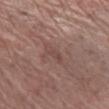Assessment:
This lesion was catalogued during total-body skin photography and was not selected for biopsy.
Clinical summary:
A male subject in their 70s. Imaged with white-light lighting. Approximately 3.5 mm at its widest. Located on the right lower leg. A 15 mm close-up extracted from a 3D total-body photography capture.